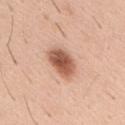- workup — total-body-photography surveillance lesion; no biopsy
- imaging modality — 15 mm crop, total-body photography
- site — the mid back
- subject — male, in their 40s